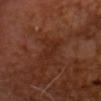The lesion was tiled from a total-body skin photograph and was not biopsied.
The lesion's longest dimension is about 4 mm.
A male patient, approximately 65 years of age.
An algorithmic analysis of the crop reported an outline eccentricity of about 0.95 (0 = round, 1 = elongated) and a symmetry-axis asymmetry near 0.35. The software also gave border irregularity of about 4.5 on a 0–10 scale, a color-variation rating of about 1/10, and peripheral color asymmetry of about 0.5. It also reported an automated nevus-likeness rating near 0 out of 100 and a lesion-detection confidence of about 90/100.
A region of skin cropped from a whole-body photographic capture, roughly 15 mm wide.
Imaged with cross-polarized lighting.